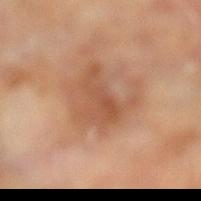Impression: Recorded during total-body skin imaging; not selected for excision or biopsy. Acquisition and patient details: A close-up tile cropped from a whole-body skin photograph, about 15 mm across. The lesion's longest dimension is about 4.5 mm. Located on the left lower leg. Automated tile analysis of the lesion measured an average lesion color of about L≈51 a*≈22 b*≈33 (CIELAB), a lesion–skin lightness drop of about 8, and a lesion-to-skin contrast of about 6 (normalized; higher = more distinct). And it measured a classifier nevus-likeness of about 0/100 and lesion-presence confidence of about 100/100. This is a cross-polarized tile. A male subject, aged approximately 65.